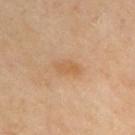The lesion was photographed on a routine skin check and not biopsied; there is no pathology result.
An algorithmic analysis of the crop reported an average lesion color of about L≈61 a*≈20 b*≈38 (CIELAB), about 7 CIELAB-L* units darker than the surrounding skin, and a normalized border contrast of about 5.5.
Longest diameter approximately 3 mm.
A roughly 15 mm field-of-view crop from a total-body skin photograph.
The lesion is on the right upper arm.
The tile uses cross-polarized illumination.